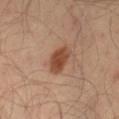workup: catalogued during a skin exam; not biopsied
illumination: cross-polarized
diameter: ~3.5 mm (longest diameter)
body site: the leg
acquisition: total-body-photography crop, ~15 mm field of view
subject: male, about 40 years old